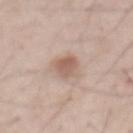biopsy status = total-body-photography surveillance lesion; no biopsy
image source = total-body-photography crop, ~15 mm field of view
lesion diameter = ~3 mm (longest diameter)
subject = male, in their 60s
site = the abdomen